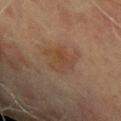Case summary:
– follow-up · catalogued during a skin exam; not biopsied
– size · ~5 mm (longest diameter)
– tile lighting · cross-polarized illumination
– body site · the left upper arm
– image source · ~15 mm tile from a whole-body skin photo
– patient · male, aged 68 to 72